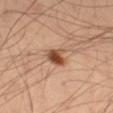The lesion was tiled from a total-body skin photograph and was not biopsied. Longest diameter approximately 2.5 mm. A 15 mm close-up extracted from a 3D total-body photography capture. Imaged with cross-polarized lighting. The lesion is located on the left thigh. A male subject about 65 years old.